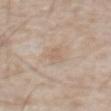Clinical summary:
A male subject, about 65 years old. Automated tile analysis of the lesion measured an area of roughly 3 mm², a shape eccentricity near 0.9, and a symmetry-axis asymmetry near 0.35. It also reported a mean CIELAB color near L≈62 a*≈14 b*≈28, a lesion–skin lightness drop of about 6, and a lesion-to-skin contrast of about 4.5 (normalized; higher = more distinct). And it measured a border-irregularity rating of about 4/10, internal color variation of about 0 on a 0–10 scale, and a peripheral color-asymmetry measure near 0. The lesion is on the abdomen. The recorded lesion diameter is about 3 mm. This image is a 15 mm lesion crop taken from a total-body photograph. The tile uses white-light illumination.